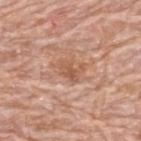patient = male, about 80 years old | tile lighting = white-light illumination | imaging modality = total-body-photography crop, ~15 mm field of view | diameter = ~3 mm (longest diameter) | location = the upper back.